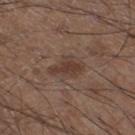Part of a total-body skin-imaging series; this lesion was reviewed on a skin check and was not flagged for biopsy.
A region of skin cropped from a whole-body photographic capture, roughly 15 mm wide.
Captured under white-light illumination.
The recorded lesion diameter is about 4 mm.
Automated tile analysis of the lesion measured a lesion area of about 7 mm², an eccentricity of roughly 0.8, and a symmetry-axis asymmetry near 0.35. The analysis additionally found a within-lesion color-variation index near 2/10 and peripheral color asymmetry of about 0.5. The analysis additionally found an automated nevus-likeness rating near 5 out of 100.
The patient is a male in their 60s.
From the left lower leg.